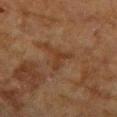Impression: No biopsy was performed on this lesion — it was imaged during a full skin examination and was not determined to be concerning. Image and clinical context: The lesion is located on the right forearm. A roughly 15 mm field-of-view crop from a total-body skin photograph. The total-body-photography lesion software estimated an eccentricity of roughly 0.55. The software also gave a classifier nevus-likeness of about 0/100. Approximately 3.5 mm at its widest. The tile uses cross-polarized illumination. The subject is a female aged around 80.